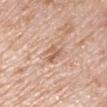The lesion was tiled from a total-body skin photograph and was not biopsied. Automated image analysis of the tile measured a classifier nevus-likeness of about 0/100 and a detector confidence of about 100 out of 100 that the crop contains a lesion. A close-up tile cropped from a whole-body skin photograph, about 15 mm across. Longest diameter approximately 3 mm. A male patient, aged 68–72. Captured under white-light illumination. Located on the right upper arm.